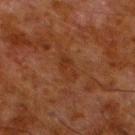{"biopsy_status": "not biopsied; imaged during a skin examination", "lesion_size": {"long_diameter_mm_approx": 3.5}, "image": {"source": "total-body photography crop", "field_of_view_mm": 15}, "site": "left lower leg", "patient": {"sex": "male", "age_approx": 80}, "automated_metrics": {"area_mm2_approx": 5.0, "eccentricity": 0.9, "cielab_L": 26, "cielab_a": 19, "cielab_b": 27, "vs_skin_darker_L": 4.0, "vs_skin_contrast_norm": 5.5, "color_variation_0_10": 2.5, "peripheral_color_asymmetry": 1.0, "nevus_likeness_0_100": 0, "lesion_detection_confidence_0_100": 100}}